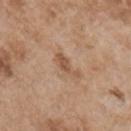* notes: total-body-photography surveillance lesion; no biopsy
* acquisition: 15 mm crop, total-body photography
* patient: male, aged 53–57
* anatomic site: the chest
* diameter: ≈3.5 mm